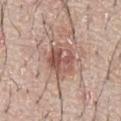This lesion was catalogued during total-body skin photography and was not selected for biopsy. A roughly 15 mm field-of-view crop from a total-body skin photograph. A male patient aged 63–67. The lesion is on the chest. Captured under white-light illumination. Measured at roughly 3.5 mm in maximum diameter.